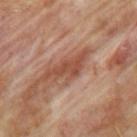notes: catalogued during a skin exam; not biopsied
TBP lesion metrics: an eccentricity of roughly 0.9 and a symmetry-axis asymmetry near 0.5; border irregularity of about 6.5 on a 0–10 scale and a peripheral color-asymmetry measure near 0.5
location: the left upper arm
patient: male, aged around 65
image: total-body-photography crop, ~15 mm field of view
lighting: cross-polarized illumination
lesion size: about 5 mm